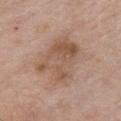The lesion was tiled from a total-body skin photograph and was not biopsied. Longest diameter approximately 6.5 mm. The lesion is located on the chest. The tile uses white-light illumination. A 15 mm close-up extracted from a 3D total-body photography capture. A male subject, aged 53–57. Automated image analysis of the tile measured an outline eccentricity of about 0.6 (0 = round, 1 = elongated) and a symmetry-axis asymmetry near 0.2. It also reported a border-irregularity rating of about 3/10, a within-lesion color-variation index near 5.5/10, and radial color variation of about 2.